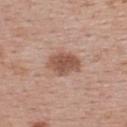This lesion was catalogued during total-body skin photography and was not selected for biopsy. Automated tile analysis of the lesion measured a lesion area of about 9 mm², an eccentricity of roughly 0.75, and a shape-asymmetry score of about 0.2 (0 = symmetric). It also reported a border-irregularity rating of about 2/10 and a color-variation rating of about 3/10. It also reported a nevus-likeness score of about 75/100 and a detector confidence of about 100 out of 100 that the crop contains a lesion. Captured under white-light illumination. Located on the back. The subject is a female aged 53 to 57. A 15 mm close-up tile from a total-body photography series done for melanoma screening.